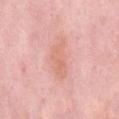follow-up = total-body-photography surveillance lesion; no biopsy | patient = male, in their mid-50s | automated metrics = a lesion area of about 10 mm² and an eccentricity of roughly 0.9; a lesion color around L≈68 a*≈25 b*≈29 in CIELAB, about 7 CIELAB-L* units darker than the surrounding skin, and a normalized border contrast of about 5; a border-irregularity index near 4.5/10, a color-variation rating of about 2.5/10, and a peripheral color-asymmetry measure near 1 | imaging modality = ~15 mm tile from a whole-body skin photo | anatomic site = the mid back.